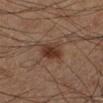notes: imaged on a skin check; not biopsied
illumination: cross-polarized
TBP lesion metrics: a classifier nevus-likeness of about 95/100
subject: male, aged approximately 60
site: the left lower leg
diameter: ~3 mm (longest diameter)
image source: 15 mm crop, total-body photography